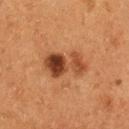Clinical impression:
Part of a total-body skin-imaging series; this lesion was reviewed on a skin check and was not flagged for biopsy.